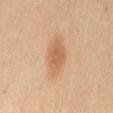Q: Was this lesion biopsied?
A: total-body-photography surveillance lesion; no biopsy
Q: Patient demographics?
A: female, aged around 45
Q: What is the anatomic site?
A: the mid back
Q: How was this image acquired?
A: ~15 mm crop, total-body skin-cancer survey